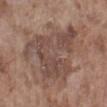location=the left lower leg | subject=female, in their mid-80s or older | lesion size=~9 mm (longest diameter) | image source=total-body-photography crop, ~15 mm field of view.